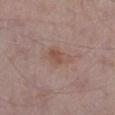{
  "biopsy_status": "not biopsied; imaged during a skin examination",
  "site": "left lower leg",
  "image": {
    "source": "total-body photography crop",
    "field_of_view_mm": 15
  },
  "lighting": "white-light",
  "patient": {
    "sex": "male",
    "age_approx": 55
  },
  "automated_metrics": {
    "eccentricity": 0.8,
    "shape_asymmetry": 0.3,
    "vs_skin_darker_L": 7.0,
    "vs_skin_contrast_norm": 6.0
  },
  "lesion_size": {
    "long_diameter_mm_approx": 4.0
  }
}